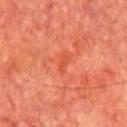follow-up: total-body-photography surveillance lesion; no biopsy | lighting: cross-polarized | anatomic site: the front of the torso | automated lesion analysis: an eccentricity of roughly 0.95 and a symmetry-axis asymmetry near 0.3; an average lesion color of about L≈49 a*≈36 b*≈39 (CIELAB), about 6 CIELAB-L* units darker than the surrounding skin, and a normalized lesion–skin contrast near 5; a within-lesion color-variation index near 0/10 and peripheral color asymmetry of about 0 | size: about 2.5 mm | image source: 15 mm crop, total-body photography | subject: male, aged approximately 65.